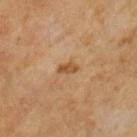{
  "biopsy_status": "not biopsied; imaged during a skin examination",
  "patient": {
    "sex": "male",
    "age_approx": 65
  },
  "image": {
    "source": "total-body photography crop",
    "field_of_view_mm": 15
  }
}